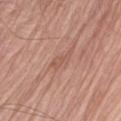workup: no biopsy performed (imaged during a skin exam) | subject: male, in their mid- to late 70s | imaging modality: ~15 mm tile from a whole-body skin photo | body site: the arm.